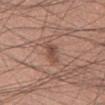<tbp_lesion>
<site>chest</site>
<lesion_size>
  <long_diameter_mm_approx>3.0</long_diameter_mm_approx>
</lesion_size>
<image>
  <source>total-body photography crop</source>
  <field_of_view_mm>15</field_of_view_mm>
</image>
<patient>
  <sex>male</sex>
  <age_approx>60</age_approx>
</patient>
</tbp_lesion>